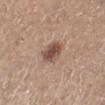Notes:
* biopsy status: catalogued during a skin exam; not biopsied
* site: the right lower leg
* lighting: white-light
* imaging modality: 15 mm crop, total-body photography
* subject: female, in their 60s
* lesion diameter: ≈3 mm
* image-analysis metrics: an area of roughly 6.5 mm², an eccentricity of roughly 0.5, and a symmetry-axis asymmetry near 0.15